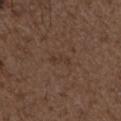Imaged during a routine full-body skin examination; the lesion was not biopsied and no histopathology is available. A region of skin cropped from a whole-body photographic capture, roughly 15 mm wide. An algorithmic analysis of the crop reported border irregularity of about 4 on a 0–10 scale and a peripheral color-asymmetry measure near 0. On the arm. The recorded lesion diameter is about 2.5 mm. A male patient, aged 48–52. Captured under white-light illumination.